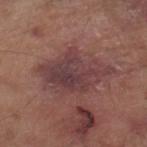| feature | finding |
|---|---|
| diameter | about 7 mm |
| acquisition | 15 mm crop, total-body photography |
| lighting | white-light |
| subject | male, aged 78 to 82 |
| location | the leg |